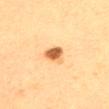follow-up: imaged on a skin check; not biopsied | patient: female, aged 28–32 | imaging modality: ~15 mm tile from a whole-body skin photo | location: the upper back.